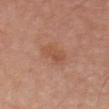* biopsy status: imaged on a skin check; not biopsied
* subject: male, roughly 70 years of age
* tile lighting: white-light illumination
* image: ~15 mm tile from a whole-body skin photo
* body site: the chest
* image-analysis metrics: a shape eccentricity near 0.9 and a symmetry-axis asymmetry near 0.25; a nevus-likeness score of about 5/100 and lesion-presence confidence of about 100/100